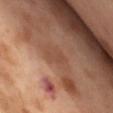Impression: Part of a total-body skin-imaging series; this lesion was reviewed on a skin check and was not flagged for biopsy. Background: The patient is a female in their mid-50s. The tile uses cross-polarized illumination. From the front of the torso. Automated tile analysis of the lesion measured an average lesion color of about L≈49 a*≈21 b*≈31 (CIELAB), roughly 6 lightness units darker than nearby skin, and a normalized lesion–skin contrast near 4.5. And it measured a border-irregularity index near 3/10 and a peripheral color-asymmetry measure near 0.5. The analysis additionally found an automated nevus-likeness rating near 0 out of 100 and a detector confidence of about 100 out of 100 that the crop contains a lesion. A 15 mm close-up tile from a total-body photography series done for melanoma screening.